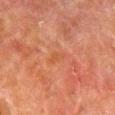Notes:
• follow-up — no biopsy performed (imaged during a skin exam)
• lighting — cross-polarized illumination
• patient — male, roughly 80 years of age
• TBP lesion metrics — a mean CIELAB color near L≈43 a*≈23 b*≈32, a lesion–skin lightness drop of about 4, and a lesion-to-skin contrast of about 4.5 (normalized; higher = more distinct); an automated nevus-likeness rating near 0 out of 100
• site — the right lower leg
• size — about 3 mm
• imaging modality — total-body-photography crop, ~15 mm field of view